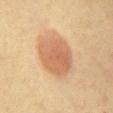Part of a total-body skin-imaging series; this lesion was reviewed on a skin check and was not flagged for biopsy. A 15 mm close-up tile from a total-body photography series done for melanoma screening. The lesion is located on the chest. The subject is a female aged 48–52.